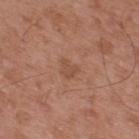Background:
A male patient, aged approximately 55. From the back. Approximately 2.5 mm at its widest. Cropped from a whole-body photographic skin survey; the tile spans about 15 mm.